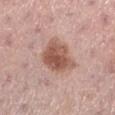{"biopsy_status": "not biopsied; imaged during a skin examination", "patient": {"sex": "female", "age_approx": 55}, "site": "left lower leg", "lighting": "white-light", "image": {"source": "total-body photography crop", "field_of_view_mm": 15}}